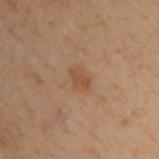Case summary:
– lighting · cross-polarized illumination
– TBP lesion metrics · an area of roughly 3 mm², an eccentricity of roughly 0.8, and a shape-asymmetry score of about 0.35 (0 = symmetric)
– location · the back
– subject · male, aged approximately 50
– imaging modality · total-body-photography crop, ~15 mm field of view